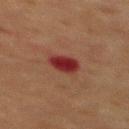imaging modality = ~15 mm tile from a whole-body skin photo
location = the mid back
patient = male, aged 48–52